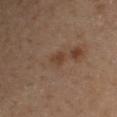The lesion was photographed on a routine skin check and not biopsied; there is no pathology result. A roughly 15 mm field-of-view crop from a total-body skin photograph. Captured under cross-polarized illumination. The lesion-visualizer software estimated an average lesion color of about L≈32 a*≈15 b*≈24 (CIELAB) and a normalized border contrast of about 6.5. The analysis additionally found a border-irregularity rating of about 3/10 and radial color variation of about 0.5. The analysis additionally found an automated nevus-likeness rating near 10 out of 100. The lesion's longest dimension is about 2.5 mm. The lesion is on the left arm. The patient is a female in their mid- to late 50s.